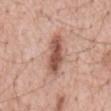Q: Is there a histopathology result?
A: total-body-photography surveillance lesion; no biopsy
Q: Patient demographics?
A: male, approximately 60 years of age
Q: Lesion location?
A: the mid back
Q: How was the tile lit?
A: white-light
Q: Lesion size?
A: ~5.5 mm (longest diameter)
Q: What did automated image analysis measure?
A: an outline eccentricity of about 0.95 (0 = round, 1 = elongated) and two-axis asymmetry of about 0.2; a lesion color around L≈54 a*≈23 b*≈27 in CIELAB, a lesion–skin lightness drop of about 14, and a normalized border contrast of about 9.5
Q: What is the imaging modality?
A: total-body-photography crop, ~15 mm field of view